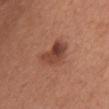{"biopsy_status": "not biopsied; imaged during a skin examination", "site": "chest", "lesion_size": {"long_diameter_mm_approx": 4.0}, "patient": {"sex": "female", "age_approx": 65}, "image": {"source": "total-body photography crop", "field_of_view_mm": 15}}